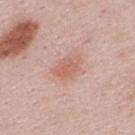The lesion was tiled from a total-body skin photograph and was not biopsied.
A 15 mm crop from a total-body photograph taken for skin-cancer surveillance.
Located on the back.
A male patient, about 25 years old.
The tile uses white-light illumination.
The recorded lesion diameter is about 4 mm.